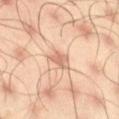Assessment: Part of a total-body skin-imaging series; this lesion was reviewed on a skin check and was not flagged for biopsy. Acquisition and patient details: A male patient roughly 45 years of age. On the right thigh. Approximately 2.5 mm at its widest. A 15 mm crop from a total-body photograph taken for skin-cancer surveillance. Captured under cross-polarized illumination.